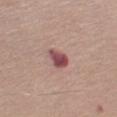On the left upper arm. Approximately 3 mm at its widest. Captured under white-light illumination. A 15 mm close-up extracted from a 3D total-body photography capture. The subject is a male aged around 75.